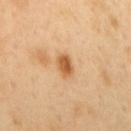{"biopsy_status": "not biopsied; imaged during a skin examination", "site": "upper back", "lesion_size": {"long_diameter_mm_approx": 3.0}, "image": {"source": "total-body photography crop", "field_of_view_mm": 15}, "patient": {"sex": "male", "age_approx": 50}, "lighting": "cross-polarized"}